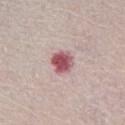Q: Was a biopsy performed?
A: imaged on a skin check; not biopsied
Q: How was this image acquired?
A: ~15 mm tile from a whole-body skin photo
Q: Where on the body is the lesion?
A: the abdomen
Q: What are the patient's age and sex?
A: male, aged around 65